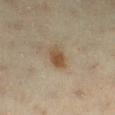Recorded during total-body skin imaging; not selected for excision or biopsy. Captured under cross-polarized illumination. Longest diameter approximately 2.5 mm. A 15 mm close-up extracted from a 3D total-body photography capture. Located on the left lower leg. An algorithmic analysis of the crop reported a footprint of about 5 mm² and two-axis asymmetry of about 0.2. And it measured an automated nevus-likeness rating near 95 out of 100. A female patient, aged 63 to 67.